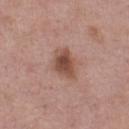Measured at roughly 3.5 mm in maximum diameter.
On the left thigh.
A female patient, roughly 65 years of age.
A 15 mm crop from a total-body photograph taken for skin-cancer surveillance.